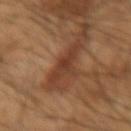| field | value |
|---|---|
| notes | catalogued during a skin exam; not biopsied |
| subject | male, roughly 65 years of age |
| image source | total-body-photography crop, ~15 mm field of view |
| anatomic site | the right forearm |
| tile lighting | cross-polarized illumination |